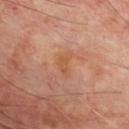<record>
<patient>
  <sex>male</sex>
  <age_approx>65</age_approx>
</patient>
<site>chest</site>
<image>
  <source>total-body photography crop</source>
  <field_of_view_mm>15</field_of_view_mm>
</image>
<lighting>cross-polarized</lighting>
<automated_metrics>
  <area_mm2_approx>3.5</area_mm2_approx>
  <nevus_likeness_0_100>0</nevus_likeness_0_100>
  <lesion_detection_confidence_0_100>100</lesion_detection_confidence_0_100>
</automated_metrics>
<lesion_size>
  <long_diameter_mm_approx>3.0</long_diameter_mm_approx>
</lesion_size>
</record>